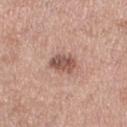This lesion was catalogued during total-body skin photography and was not selected for biopsy. An algorithmic analysis of the crop reported a footprint of about 6 mm², a shape eccentricity near 0.65, and two-axis asymmetry of about 0.2. The analysis additionally found a lesion color around L≈53 a*≈21 b*≈25 in CIELAB and roughly 13 lightness units darker than nearby skin. It also reported border irregularity of about 2 on a 0–10 scale, a color-variation rating of about 3.5/10, and a peripheral color-asymmetry measure near 1.5. It also reported a lesion-detection confidence of about 100/100. A region of skin cropped from a whole-body photographic capture, roughly 15 mm wide. Measured at roughly 3 mm in maximum diameter. On the left thigh. The patient is a female about 40 years old. This is a white-light tile.